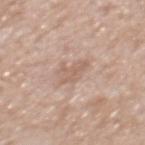Part of a total-body skin-imaging series; this lesion was reviewed on a skin check and was not flagged for biopsy.
A male subject, aged around 65.
The lesion is located on the mid back.
A 15 mm close-up tile from a total-body photography series done for melanoma screening.